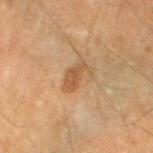No biopsy was performed on this lesion — it was imaged during a full skin examination and was not determined to be concerning. Located on the left lower leg. Cropped from a whole-body photographic skin survey; the tile spans about 15 mm. A male subject, aged 48–52. This is a cross-polarized tile.